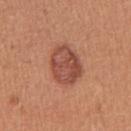Q: Was a biopsy performed?
A: no biopsy performed (imaged during a skin exam)
Q: What is the lesion's diameter?
A: ~5 mm (longest diameter)
Q: What are the patient's age and sex?
A: female, aged around 40
Q: What lighting was used for the tile?
A: white-light
Q: What is the anatomic site?
A: the arm
Q: How was this image acquired?
A: total-body-photography crop, ~15 mm field of view